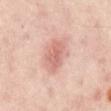biopsy status: no biopsy performed (imaged during a skin exam)
image: ~15 mm tile from a whole-body skin photo
anatomic site: the abdomen
subject: about 55 years old
lesion size: ~4 mm (longest diameter)
image-analysis metrics: an average lesion color of about L≈64 a*≈24 b*≈26 (CIELAB), a lesion–skin lightness drop of about 10, and a normalized lesion–skin contrast near 6; a border-irregularity index near 2/10, a within-lesion color-variation index near 3/10, and a peripheral color-asymmetry measure near 1